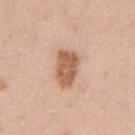Assessment: Recorded during total-body skin imaging; not selected for excision or biopsy. Context: The lesion is located on the front of the torso. Cropped from a total-body skin-imaging series; the visible field is about 15 mm. Longest diameter approximately 4.5 mm. A male patient, about 45 years old.